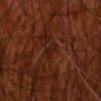This lesion was catalogued during total-body skin photography and was not selected for biopsy.
This is a cross-polarized tile.
A male subject, aged 68–72.
A close-up tile cropped from a whole-body skin photograph, about 15 mm across.
The lesion-visualizer software estimated a shape eccentricity near 0.9 and a shape-asymmetry score of about 0.35 (0 = symmetric). The analysis additionally found a detector confidence of about 65 out of 100 that the crop contains a lesion.
On the right upper arm.
The lesion's longest dimension is about 3 mm.